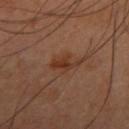Recorded during total-body skin imaging; not selected for excision or biopsy.
The subject is a male roughly 45 years of age.
Longest diameter approximately 3 mm.
Imaged with cross-polarized lighting.
On the right upper arm.
A 15 mm close-up tile from a total-body photography series done for melanoma screening.